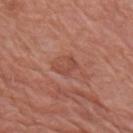No biopsy was performed on this lesion — it was imaged during a full skin examination and was not determined to be concerning.
The lesion is located on the right upper arm.
Cropped from a total-body skin-imaging series; the visible field is about 15 mm.
A male patient, in their 80s.
Captured under white-light illumination.
The lesion's longest dimension is about 3 mm.
Automated image analysis of the tile measured about 7 CIELAB-L* units darker than the surrounding skin and a lesion-to-skin contrast of about 5 (normalized; higher = more distinct). It also reported an automated nevus-likeness rating near 0 out of 100 and a lesion-detection confidence of about 100/100.